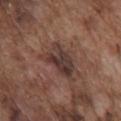{
  "patient": {
    "sex": "male",
    "age_approx": 75
  },
  "image": {
    "source": "total-body photography crop",
    "field_of_view_mm": 15
  },
  "lighting": "white-light",
  "site": "chest",
  "automated_metrics": {
    "area_mm2_approx": 12.0,
    "eccentricity": 0.8,
    "vs_skin_darker_L": 9.0,
    "color_variation_0_10": 6.5,
    "peripheral_color_asymmetry": 2.5,
    "nevus_likeness_0_100": 0
  },
  "lesion_size": {
    "long_diameter_mm_approx": 5.0
  }
}